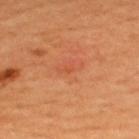biopsy status: no biopsy performed (imaged during a skin exam); location: the upper back; patient: male, aged 63 to 67; automated metrics: border irregularity of about 3.5 on a 0–10 scale, internal color variation of about 0 on a 0–10 scale, and a peripheral color-asymmetry measure near 0; image: ~15 mm crop, total-body skin-cancer survey; lesion diameter: ~1 mm (longest diameter); illumination: cross-polarized.